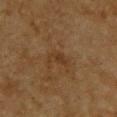Acquisition and patient details: A male subject, approximately 85 years of age or older. A 15 mm crop from a total-body photograph taken for skin-cancer surveillance. On the upper back.